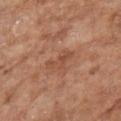Clinical impression: Recorded during total-body skin imaging; not selected for excision or biopsy. Acquisition and patient details: A female subject aged 73–77. Automated tile analysis of the lesion measured a mean CIELAB color near L≈49 a*≈23 b*≈32, about 7 CIELAB-L* units darker than the surrounding skin, and a lesion-to-skin contrast of about 5.5 (normalized; higher = more distinct). The lesion's longest dimension is about 3.5 mm. The lesion is on the arm. A lesion tile, about 15 mm wide, cut from a 3D total-body photograph.